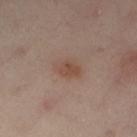Assessment: The lesion was tiled from a total-body skin photograph and was not biopsied. Context: Automated image analysis of the tile measured an area of roughly 7 mm², a shape eccentricity near 0.7, and a shape-asymmetry score of about 0.15 (0 = symmetric). The analysis additionally found a lesion color around L≈49 a*≈18 b*≈26 in CIELAB, about 7 CIELAB-L* units darker than the surrounding skin, and a lesion-to-skin contrast of about 6 (normalized; higher = more distinct). It also reported a border-irregularity index near 1.5/10 and internal color variation of about 4 on a 0–10 scale. The software also gave an automated nevus-likeness rating near 70 out of 100 and a detector confidence of about 100 out of 100 that the crop contains a lesion. A female patient, aged approximately 55. The tile uses cross-polarized illumination. A region of skin cropped from a whole-body photographic capture, roughly 15 mm wide. On the left thigh.